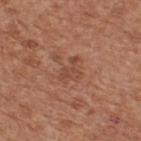The lesion was tiled from a total-body skin photograph and was not biopsied.
The recorded lesion diameter is about 3.5 mm.
This is a white-light tile.
Located on the upper back.
A 15 mm close-up extracted from a 3D total-body photography capture.
A male subject, approximately 65 years of age.
An algorithmic analysis of the crop reported a lesion–skin lightness drop of about 7. And it measured a nevus-likeness score of about 0/100 and a lesion-detection confidence of about 100/100.